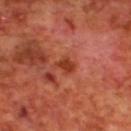Clinical impression:
This lesion was catalogued during total-body skin photography and was not selected for biopsy.
Acquisition and patient details:
A roughly 15 mm field-of-view crop from a total-body skin photograph. Imaged with cross-polarized lighting. A male subject, aged approximately 70. Located on the upper back. The lesion-visualizer software estimated a shape-asymmetry score of about 0.35 (0 = symmetric). It also reported a mean CIELAB color near L≈39 a*≈31 b*≈35, roughly 9 lightness units darker than nearby skin, and a normalized border contrast of about 8. And it measured internal color variation of about 3 on a 0–10 scale and a peripheral color-asymmetry measure near 1. It also reported a classifier nevus-likeness of about 0/100 and a lesion-detection confidence of about 100/100. Measured at roughly 2.5 mm in maximum diameter.